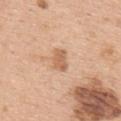Assessment:
Imaged during a routine full-body skin examination; the lesion was not biopsied and no histopathology is available.
Clinical summary:
From the upper back. The subject is a female aged 63 to 67. Approximately 3.5 mm at its widest. A 15 mm close-up extracted from a 3D total-body photography capture.